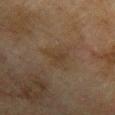Clinical impression: Part of a total-body skin-imaging series; this lesion was reviewed on a skin check and was not flagged for biopsy. Context: Cropped from a whole-body photographic skin survey; the tile spans about 15 mm. Measured at roughly 3.5 mm in maximum diameter. A male patient, about 75 years old. From the left upper arm. This is a cross-polarized tile.